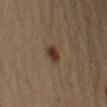Clinical impression: The lesion was photographed on a routine skin check and not biopsied; there is no pathology result. Acquisition and patient details: The recorded lesion diameter is about 2.5 mm. The lesion is located on the arm. A male patient, aged approximately 60. Cropped from a total-body skin-imaging series; the visible field is about 15 mm.